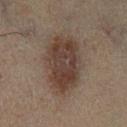Findings:
- workup: total-body-photography surveillance lesion; no biopsy
- patient: male, aged approximately 70
- anatomic site: the leg
- imaging modality: ~15 mm tile from a whole-body skin photo